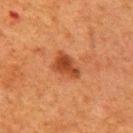Impression:
No biopsy was performed on this lesion — it was imaged during a full skin examination and was not determined to be concerning.
Background:
The patient is a female approximately 50 years of age. The total-body-photography lesion software estimated border irregularity of about 3 on a 0–10 scale and radial color variation of about 1. The analysis additionally found a nevus-likeness score of about 90/100 and lesion-presence confidence of about 100/100. A region of skin cropped from a whole-body photographic capture, roughly 15 mm wide. Captured under cross-polarized illumination. The lesion is located on the right upper arm.